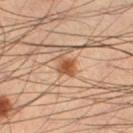Recorded during total-body skin imaging; not selected for excision or biopsy. Imaged with cross-polarized lighting. The subject is a male in their mid- to late 50s. Measured at roughly 3.5 mm in maximum diameter. Located on the leg. A 15 mm close-up tile from a total-body photography series done for melanoma screening.